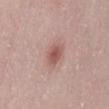The lesion is located on the abdomen. Longest diameter approximately 3 mm. A roughly 15 mm field-of-view crop from a total-body skin photograph. An algorithmic analysis of the crop reported a shape eccentricity near 0.6 and a symmetry-axis asymmetry near 0.25. And it measured a mean CIELAB color near L≈55 a*≈23 b*≈24, about 11 CIELAB-L* units darker than the surrounding skin, and a lesion-to-skin contrast of about 7.5 (normalized; higher = more distinct). It also reported a detector confidence of about 100 out of 100 that the crop contains a lesion. A female subject approximately 30 years of age.